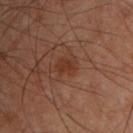Q: Was a biopsy performed?
A: imaged on a skin check; not biopsied
Q: What kind of image is this?
A: ~15 mm crop, total-body skin-cancer survey
Q: Where on the body is the lesion?
A: the chest
Q: Lesion size?
A: ≈2.5 mm
Q: How was the tile lit?
A: cross-polarized illumination
Q: What are the patient's age and sex?
A: male, approximately 50 years of age